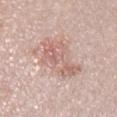Assessment: The lesion was tiled from a total-body skin photograph and was not biopsied. Acquisition and patient details: A lesion tile, about 15 mm wide, cut from a 3D total-body photograph. This is a white-light tile. The recorded lesion diameter is about 6 mm. The lesion-visualizer software estimated an area of roughly 16 mm² and two-axis asymmetry of about 0.3. It also reported a border-irregularity rating of about 4/10, internal color variation of about 5 on a 0–10 scale, and radial color variation of about 1.5. A male subject, aged 28 to 32.